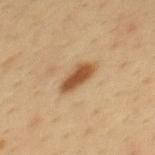| feature | finding |
|---|---|
| workup | imaged on a skin check; not biopsied |
| lesion size | ~4 mm (longest diameter) |
| image source | ~15 mm tile from a whole-body skin photo |
| patient | male, about 35 years old |
| body site | the back |
| tile lighting | cross-polarized illumination |